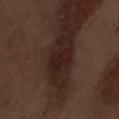Part of a total-body skin-imaging series; this lesion was reviewed on a skin check and was not flagged for biopsy. Automated tile analysis of the lesion measured an average lesion color of about L≈20 a*≈18 b*≈18 (CIELAB), roughly 7 lightness units darker than nearby skin, and a normalized border contrast of about 8.5. The software also gave a detector confidence of about 50 out of 100 that the crop contains a lesion. This is a white-light tile. Longest diameter approximately 3 mm. A male patient, about 70 years old. On the abdomen. A close-up tile cropped from a whole-body skin photograph, about 15 mm across.